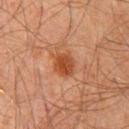{
  "biopsy_status": "not biopsied; imaged during a skin examination",
  "patient": {
    "sex": "male",
    "age_approx": 60
  },
  "lesion_size": {
    "long_diameter_mm_approx": 3.0
  },
  "lighting": "cross-polarized",
  "image": {
    "source": "total-body photography crop",
    "field_of_view_mm": 15
  },
  "site": "chest"
}